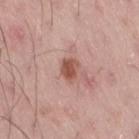Findings:
* notes — catalogued during a skin exam; not biopsied
* patient — male, in their 50s
* image — total-body-photography crop, ~15 mm field of view
* location — the right thigh
* lesion diameter — about 2.5 mm